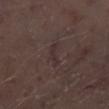Imaged during a routine full-body skin examination; the lesion was not biopsied and no histopathology is available.
A male subject, aged 68 to 72.
A 15 mm close-up extracted from a 3D total-body photography capture.
The lesion is on the left lower leg.